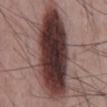Notes:
- biopsy status · total-body-photography surveillance lesion; no biopsy
- body site · the back
- patient · male, in their mid- to late 50s
- imaging modality · 15 mm crop, total-body photography
- lesion diameter · ~12.5 mm (longest diameter)